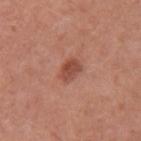Findings:
– follow-up: catalogued during a skin exam; not biopsied
– image-analysis metrics: an average lesion color of about L≈48 a*≈26 b*≈29 (CIELAB), roughly 10 lightness units darker than nearby skin, and a normalized lesion–skin contrast near 7.5; a border-irregularity rating of about 2/10 and peripheral color asymmetry of about 0.5; an automated nevus-likeness rating near 80 out of 100 and a lesion-detection confidence of about 100/100
– location: the arm
– illumination: white-light
– imaging modality: ~15 mm tile from a whole-body skin photo
– size: ~3 mm (longest diameter)
– patient: female, in their mid- to late 30s